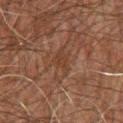notes: imaged on a skin check; not biopsied
image: 15 mm crop, total-body photography
subject: male, aged around 60
site: the chest
size: ~3 mm (longest diameter)